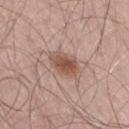Q: Is there a histopathology result?
A: imaged on a skin check; not biopsied
Q: Illumination type?
A: white-light
Q: Where on the body is the lesion?
A: the right thigh
Q: How large is the lesion?
A: ~3.5 mm (longest diameter)
Q: What are the patient's age and sex?
A: male, aged around 70
Q: What kind of image is this?
A: ~15 mm tile from a whole-body skin photo